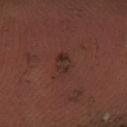follow-up = catalogued during a skin exam; not biopsied
anatomic site = the right forearm
TBP lesion metrics = a symmetry-axis asymmetry near 0.2; a classifier nevus-likeness of about 30/100 and lesion-presence confidence of about 100/100
image source = ~15 mm crop, total-body skin-cancer survey
size = ~2.5 mm (longest diameter)
patient = male, aged around 65